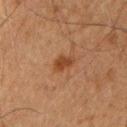Recorded during total-body skin imaging; not selected for excision or biopsy. A male patient, in their mid-60s. From the chest. A region of skin cropped from a whole-body photographic capture, roughly 15 mm wide. Imaged with cross-polarized lighting.